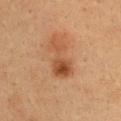Q: Was a biopsy performed?
A: total-body-photography surveillance lesion; no biopsy
Q: What are the patient's age and sex?
A: female, aged 28 to 32
Q: What kind of image is this?
A: total-body-photography crop, ~15 mm field of view
Q: Illumination type?
A: cross-polarized illumination
Q: Lesion location?
A: the back
Q: What is the lesion's diameter?
A: ≈5 mm